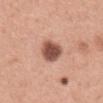Q: Is there a histopathology result?
A: no biopsy performed (imaged during a skin exam)
Q: Patient demographics?
A: female, approximately 35 years of age
Q: Illumination type?
A: white-light illumination
Q: What is the lesion's diameter?
A: ≈4.5 mm
Q: How was this image acquired?
A: 15 mm crop, total-body photography
Q: What is the anatomic site?
A: the back